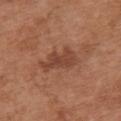biopsy_status: not biopsied; imaged during a skin examination
automated_metrics:
  eccentricity: 0.85
  shape_asymmetry: 0.35
  cielab_L: 44
  cielab_a: 23
  cielab_b: 30
  vs_skin_darker_L: 9.0
patient:
  sex: female
  age_approx: 65
image:
  source: total-body photography crop
  field_of_view_mm: 15
site: chest